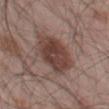subject — male, aged 53–57
lesion size — about 6 mm
body site — the leg
tile lighting — white-light illumination
image — ~15 mm tile from a whole-body skin photo